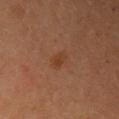Imaged during a routine full-body skin examination; the lesion was not biopsied and no histopathology is available. Located on the right upper arm. The subject is a female aged approximately 35. A 15 mm close-up tile from a total-body photography series done for melanoma screening.